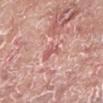Impression:
Part of a total-body skin-imaging series; this lesion was reviewed on a skin check and was not flagged for biopsy.
Context:
A male patient, roughly 65 years of age. About 2.5 mm across. This image is a 15 mm lesion crop taken from a total-body photograph. Automated tile analysis of the lesion measured an area of roughly 4 mm², an eccentricity of roughly 0.35, and a symmetry-axis asymmetry near 0.45. The analysis additionally found a lesion color around L≈59 a*≈27 b*≈25 in CIELAB and a normalized lesion–skin contrast near 5.5. And it measured a border-irregularity index near 5/10, a color-variation rating of about 2/10, and radial color variation of about 0.5. And it measured a classifier nevus-likeness of about 0/100 and a detector confidence of about 100 out of 100 that the crop contains a lesion. The tile uses white-light illumination. The lesion is on the left lower leg.